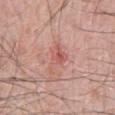workup: total-body-photography surveillance lesion; no biopsy
location: the abdomen
patient: male, roughly 60 years of age
lighting: white-light
automated metrics: a footprint of about 6.5 mm² and an eccentricity of roughly 0.8; a lesion-to-skin contrast of about 5.5 (normalized; higher = more distinct); a within-lesion color-variation index near 5/10 and peripheral color asymmetry of about 1.5
image: 15 mm crop, total-body photography